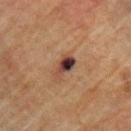follow-up=no biopsy performed (imaged during a skin exam)
lighting=cross-polarized
site=the chest
imaging modality=~15 mm crop, total-body skin-cancer survey
size=about 3.5 mm
subject=male, aged 73 to 77